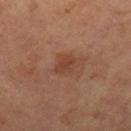The lesion was photographed on a routine skin check and not biopsied; there is no pathology result.
A female subject, in their mid-60s.
The tile uses cross-polarized illumination.
On the right thigh.
This image is a 15 mm lesion crop taken from a total-body photograph.
Approximately 2.5 mm at its widest.